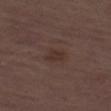Notes:
- biopsy status — imaged on a skin check; not biopsied
- location — the left thigh
- tile lighting — white-light illumination
- size — ≈3 mm
- subject — male, about 70 years old
- imaging modality — total-body-photography crop, ~15 mm field of view
- automated lesion analysis — a mean CIELAB color near L≈31 a*≈16 b*≈20, about 5 CIELAB-L* units darker than the surrounding skin, and a normalized border contrast of about 6; a border-irregularity index near 3/10, internal color variation of about 1 on a 0–10 scale, and peripheral color asymmetry of about 0.5